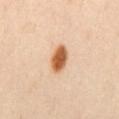No biopsy was performed on this lesion — it was imaged during a full skin examination and was not determined to be concerning. An algorithmic analysis of the crop reported a footprint of about 7 mm², an outline eccentricity of about 0.8 (0 = round, 1 = elongated), and a symmetry-axis asymmetry near 0.1. And it measured a lesion color around L≈61 a*≈23 b*≈39 in CIELAB, roughly 17 lightness units darker than nearby skin, and a normalized lesion–skin contrast near 11.5. The software also gave border irregularity of about 1 on a 0–10 scale, a color-variation rating of about 4/10, and a peripheral color-asymmetry measure near 1. The analysis additionally found a detector confidence of about 100 out of 100 that the crop contains a lesion. The lesion is on the mid back. Imaged with cross-polarized lighting. A female subject in their 40s. A lesion tile, about 15 mm wide, cut from a 3D total-body photograph.